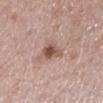<record>
<biopsy_status>not biopsied; imaged during a skin examination</biopsy_status>
<image>
  <source>total-body photography crop</source>
  <field_of_view_mm>15</field_of_view_mm>
</image>
<automated_metrics>
  <area_mm2_approx>5.0</area_mm2_approx>
  <eccentricity>0.8</eccentricity>
  <shape_asymmetry>0.3</shape_asymmetry>
  <cielab_L>52</cielab_L>
  <cielab_a>20</cielab_a>
  <cielab_b>25</cielab_b>
  <vs_skin_darker_L>13.0</vs_skin_darker_L>
  <vs_skin_contrast_norm>9.0</vs_skin_contrast_norm>
  <nevus_likeness_0_100>75</nevus_likeness_0_100>
  <lesion_detection_confidence_0_100>100</lesion_detection_confidence_0_100>
</automated_metrics>
<site>left lower leg</site>
<patient>
  <sex>female</sex>
  <age_approx>65</age_approx>
</patient>
<lighting>white-light</lighting>
</record>